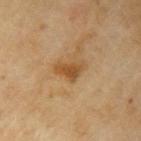Imaged during a routine full-body skin examination; the lesion was not biopsied and no histopathology is available.
A female subject about 60 years old.
Located on the left upper arm.
A close-up tile cropped from a whole-body skin photograph, about 15 mm across.